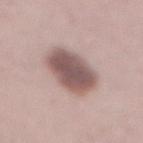Clinical impression: No biopsy was performed on this lesion — it was imaged during a full skin examination and was not determined to be concerning. Clinical summary: The tile uses white-light illumination. The lesion is on the abdomen. Automated image analysis of the tile measured a lesion color around L≈54 a*≈17 b*≈20 in CIELAB, about 16 CIELAB-L* units darker than the surrounding skin, and a normalized lesion–skin contrast near 10.5. The analysis additionally found a nevus-likeness score of about 40/100 and a detector confidence of about 100 out of 100 that the crop contains a lesion. The subject is a female about 50 years old. Cropped from a whole-body photographic skin survey; the tile spans about 15 mm.